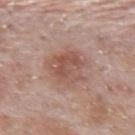Q: Lesion location?
A: the upper back
Q: Illumination type?
A: white-light
Q: Who is the patient?
A: male, roughly 55 years of age
Q: What is the imaging modality?
A: total-body-photography crop, ~15 mm field of view